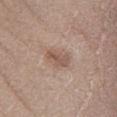No biopsy was performed on this lesion — it was imaged during a full skin examination and was not determined to be concerning.
A male patient aged 58 to 62.
From the right lower leg.
The lesion-visualizer software estimated an average lesion color of about L≈54 a*≈17 b*≈25 (CIELAB), about 9 CIELAB-L* units darker than the surrounding skin, and a lesion-to-skin contrast of about 6.5 (normalized; higher = more distinct). The software also gave border irregularity of about 3 on a 0–10 scale, internal color variation of about 3 on a 0–10 scale, and a peripheral color-asymmetry measure near 1.
The recorded lesion diameter is about 3.5 mm.
This is a white-light tile.
Cropped from a total-body skin-imaging series; the visible field is about 15 mm.